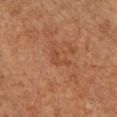biopsy status = catalogued during a skin exam; not biopsied | anatomic site = the chest | tile lighting = cross-polarized | imaging modality = total-body-photography crop, ~15 mm field of view | patient = female, approximately 50 years of age | lesion size = ~3 mm (longest diameter).